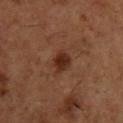notes=total-body-photography surveillance lesion; no biopsy | patient=male, aged around 55 | size=≈3 mm | tile lighting=cross-polarized illumination | site=the chest | acquisition=15 mm crop, total-body photography.